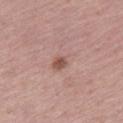workup: no biopsy performed (imaged during a skin exam)